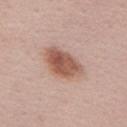Clinical impression:
This lesion was catalogued during total-body skin photography and was not selected for biopsy.
Image and clinical context:
Longest diameter approximately 5 mm. The lesion-visualizer software estimated a footprint of about 13 mm² and a shape eccentricity near 0.8. And it measured internal color variation of about 5 on a 0–10 scale. A female patient, aged 33 to 37. Cropped from a whole-body photographic skin survey; the tile spans about 15 mm. From the chest.